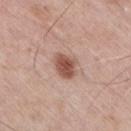{"biopsy_status": "not biopsied; imaged during a skin examination", "image": {"source": "total-body photography crop", "field_of_view_mm": 15}, "lighting": "white-light", "patient": {"sex": "male", "age_approx": 75}, "site": "right thigh"}